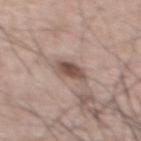Clinical impression: No biopsy was performed on this lesion — it was imaged during a full skin examination and was not determined to be concerning. Clinical summary: The tile uses white-light illumination. A male patient, aged 63 to 67. The recorded lesion diameter is about 3 mm. On the upper back. An algorithmic analysis of the crop reported a lesion area of about 5.5 mm², a shape eccentricity near 0.8, and a shape-asymmetry score of about 0.25 (0 = symmetric). It also reported border irregularity of about 2.5 on a 0–10 scale, a color-variation rating of about 3.5/10, and peripheral color asymmetry of about 1. A 15 mm close-up tile from a total-body photography series done for melanoma screening.